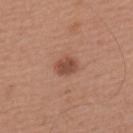Q: Was this lesion biopsied?
A: catalogued during a skin exam; not biopsied
Q: How was this image acquired?
A: ~15 mm tile from a whole-body skin photo
Q: Who is the patient?
A: male, in their mid- to late 60s
Q: How was the tile lit?
A: white-light
Q: Lesion size?
A: ~2.5 mm (longest diameter)
Q: What did automated image analysis measure?
A: a classifier nevus-likeness of about 90/100
Q: What is the anatomic site?
A: the upper back